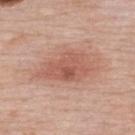Impression: This lesion was catalogued during total-body skin photography and was not selected for biopsy.